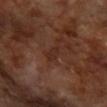Findings:
- workup: imaged on a skin check; not biopsied
- image source: 15 mm crop, total-body photography
- body site: the right forearm
- subject: female, in their mid-60s